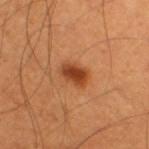| feature | finding |
|---|---|
| workup | total-body-photography surveillance lesion; no biopsy |
| lighting | cross-polarized illumination |
| subject | male, aged around 55 |
| lesion size | ~3 mm (longest diameter) |
| imaging modality | 15 mm crop, total-body photography |
| anatomic site | the leg |